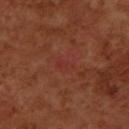Part of a total-body skin-imaging series; this lesion was reviewed on a skin check and was not flagged for biopsy.
Automated image analysis of the tile measured a footprint of about 2.5 mm², an outline eccentricity of about 0.85 (0 = round, 1 = elongated), and two-axis asymmetry of about 0.35. The software also gave a mean CIELAB color near L≈34 a*≈30 b*≈27 and a normalized lesion–skin contrast near 3. It also reported a border-irregularity rating of about 3.5/10 and radial color variation of about 0.
The lesion is located on the left forearm.
Measured at roughly 2 mm in maximum diameter.
A 15 mm close-up tile from a total-body photography series done for melanoma screening.
A female subject, aged 48 to 52.